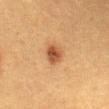{
  "biopsy_status": "not biopsied; imaged during a skin examination"
}